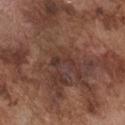Assessment: The lesion was tiled from a total-body skin photograph and was not biopsied. Clinical summary: Captured under white-light illumination. A 15 mm close-up tile from a total-body photography series done for melanoma screening. A male patient aged 73 to 77. The recorded lesion diameter is about 3 mm. An algorithmic analysis of the crop reported an area of roughly 3 mm², an eccentricity of roughly 0.9, and a symmetry-axis asymmetry near 0.45. The analysis additionally found a lesion color around L≈34 a*≈18 b*≈22 in CIELAB, a lesion–skin lightness drop of about 6, and a normalized lesion–skin contrast near 6.5. The software also gave a nevus-likeness score of about 0/100. Located on the chest.